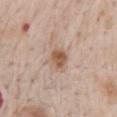Captured during whole-body skin photography for melanoma surveillance; the lesion was not biopsied. A roughly 15 mm field-of-view crop from a total-body skin photograph. The total-body-photography lesion software estimated a lesion color around L≈56 a*≈19 b*≈30 in CIELAB, roughly 11 lightness units darker than nearby skin, and a lesion-to-skin contrast of about 8.5 (normalized; higher = more distinct). The patient is a male in their mid- to late 60s. From the back. This is a white-light tile. The lesion's longest dimension is about 2.5 mm.